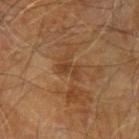This image is a 15 mm lesion crop taken from a total-body photograph.
A male subject, aged around 70.
Measured at roughly 3 mm in maximum diameter.
The lesion is located on the left upper arm.
This is a cross-polarized tile.